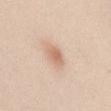| key | value |
|---|---|
| biopsy status | catalogued during a skin exam; not biopsied |
| body site | the mid back |
| size | ~3 mm (longest diameter) |
| acquisition | total-body-photography crop, ~15 mm field of view |
| patient | female, aged 23 to 27 |